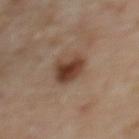follow-up: total-body-photography surveillance lesion; no biopsy | TBP lesion metrics: an area of roughly 8.5 mm² and a shape eccentricity near 0.65; a lesion color around L≈40 a*≈18 b*≈27 in CIELAB; a detector confidence of about 100 out of 100 that the crop contains a lesion | imaging modality: total-body-photography crop, ~15 mm field of view | lesion diameter: ≈3.5 mm | tile lighting: cross-polarized | subject: female, about 60 years old | location: the upper back.